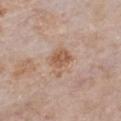This lesion was catalogued during total-body skin photography and was not selected for biopsy.
The lesion is on the chest.
This is a white-light tile.
A close-up tile cropped from a whole-body skin photograph, about 15 mm across.
A female patient roughly 85 years of age or older.